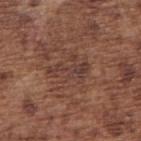Imaged during a routine full-body skin examination; the lesion was not biopsied and no histopathology is available.
This is a white-light tile.
The lesion is located on the right upper arm.
A roughly 15 mm field-of-view crop from a total-body skin photograph.
The recorded lesion diameter is about 4.5 mm.
A male patient, aged 73–77.